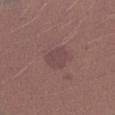Q: Lesion location?
A: the arm
Q: What kind of image is this?
A: ~15 mm tile from a whole-body skin photo
Q: Patient demographics?
A: female, aged 23–27